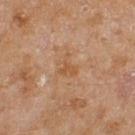{"patient": {"sex": "female", "age_approx": 60}, "site": "right thigh", "lesion_size": {"long_diameter_mm_approx": 3.0}, "image": {"source": "total-body photography crop", "field_of_view_mm": 15}, "lighting": "cross-polarized"}